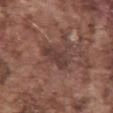The lesion was photographed on a routine skin check and not biopsied; there is no pathology result. This is a white-light tile. Cropped from a total-body skin-imaging series; the visible field is about 15 mm. A male subject aged 73 to 77. Automated tile analysis of the lesion measured an automated nevus-likeness rating near 0 out of 100 and lesion-presence confidence of about 80/100. From the abdomen.